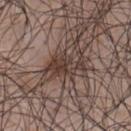A lesion tile, about 15 mm wide, cut from a 3D total-body photograph. The lesion is located on the chest. The tile uses white-light illumination. A male patient aged 43 to 47. An algorithmic analysis of the crop reported an average lesion color of about L≈38 a*≈15 b*≈21 (CIELAB), about 10 CIELAB-L* units darker than the surrounding skin, and a normalized border contrast of about 8.5. It also reported a nevus-likeness score of about 45/100 and a lesion-detection confidence of about 90/100. The recorded lesion diameter is about 5 mm.